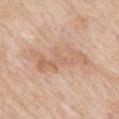workup=total-body-photography surveillance lesion; no biopsy | imaging modality=~15 mm tile from a whole-body skin photo | location=the mid back | patient=male, in their mid- to late 80s or older.